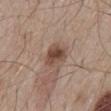Q: Was this lesion biopsied?
A: no biopsy performed (imaged during a skin exam)
Q: What lighting was used for the tile?
A: white-light
Q: What kind of image is this?
A: total-body-photography crop, ~15 mm field of view
Q: Lesion location?
A: the mid back
Q: How large is the lesion?
A: about 4 mm
Q: Who is the patient?
A: male, aged 53 to 57